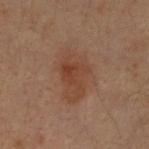This lesion was catalogued during total-body skin photography and was not selected for biopsy.
Cropped from a whole-body photographic skin survey; the tile spans about 15 mm.
From the upper back.
The lesion's longest dimension is about 6.5 mm.
The patient is a male aged 28–32.